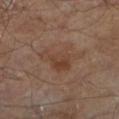A 15 mm close-up extracted from a 3D total-body photography capture. Imaged with cross-polarized lighting. Longest diameter approximately 4.5 mm. The subject is a male about 65 years old. The lesion is on the left lower leg.